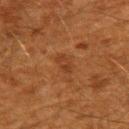workup = no biopsy performed (imaged during a skin exam)
size = ≈2.5 mm
site = the back
image source = 15 mm crop, total-body photography
TBP lesion metrics = a footprint of about 3.5 mm², a shape eccentricity near 0.8, and a symmetry-axis asymmetry near 0.2; a lesion–skin lightness drop of about 5 and a normalized lesion–skin contrast near 5.5; a within-lesion color-variation index near 2/10 and radial color variation of about 1; a lesion-detection confidence of about 100/100
subject = male, approximately 60 years of age
lighting = cross-polarized